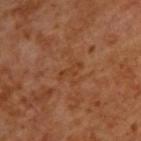– biopsy status: no biopsy performed (imaged during a skin exam)
– location: the upper back
– patient: male, aged approximately 65
– illumination: cross-polarized illumination
– image source: ~15 mm tile from a whole-body skin photo
– size: ≈3 mm